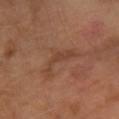Recorded during total-body skin imaging; not selected for excision or biopsy. The patient is a female about 70 years old. A 15 mm close-up extracted from a 3D total-body photography capture. Imaged with cross-polarized lighting. The lesion is on the right forearm.